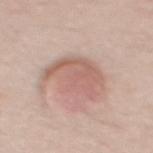follow-up: catalogued during a skin exam; not biopsied | illumination: white-light illumination | location: the upper back | patient: male, about 25 years old | diameter: ≈5.5 mm | image source: total-body-photography crop, ~15 mm field of view.